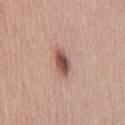Assessment:
Recorded during total-body skin imaging; not selected for excision or biopsy.